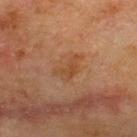The lesion was tiled from a total-body skin photograph and was not biopsied. An algorithmic analysis of the crop reported a mean CIELAB color near L≈38 a*≈18 b*≈30, a lesion–skin lightness drop of about 5, and a normalized lesion–skin contrast near 5.5. The software also gave a classifier nevus-likeness of about 0/100 and a lesion-detection confidence of about 100/100. A male subject, aged 68 to 72. A close-up tile cropped from a whole-body skin photograph, about 15 mm across. Captured under cross-polarized illumination. Measured at roughly 4 mm in maximum diameter. On the upper back.